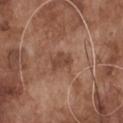The recorded lesion diameter is about 2.5 mm. The lesion is located on the chest. A male patient, aged around 75. Cropped from a total-body skin-imaging series; the visible field is about 15 mm.